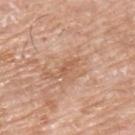follow-up — total-body-photography surveillance lesion; no biopsy | patient — male, about 75 years old | illumination — white-light | anatomic site — the upper back | lesion diameter — ≈2.5 mm | image source — ~15 mm tile from a whole-body skin photo | TBP lesion metrics — a lesion area of about 2.5 mm²; a nevus-likeness score of about 0/100 and a detector confidence of about 100 out of 100 that the crop contains a lesion.